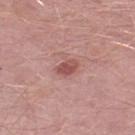- follow-up — no biopsy performed (imaged during a skin exam)
- image — 15 mm crop, total-body photography
- lighting — white-light
- lesion size — ≈2.5 mm
- location — the leg
- automated lesion analysis — a lesion–skin lightness drop of about 11 and a normalized lesion–skin contrast near 8
- patient — male, aged 48–52